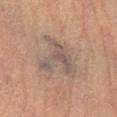Q: Was a biopsy performed?
A: imaged on a skin check; not biopsied
Q: Automated lesion metrics?
A: a footprint of about 13 mm² and two-axis asymmetry of about 0.65; a lesion color around L≈45 a*≈12 b*≈19 in CIELAB and about 7 CIELAB-L* units darker than the surrounding skin; a color-variation rating of about 3.5/10 and a peripheral color-asymmetry measure near 1; lesion-presence confidence of about 70/100
Q: What is the imaging modality?
A: total-body-photography crop, ~15 mm field of view
Q: What is the anatomic site?
A: the left lower leg
Q: What is the lesion's diameter?
A: about 5 mm
Q: What lighting was used for the tile?
A: cross-polarized
Q: Patient demographics?
A: male, about 85 years old or older